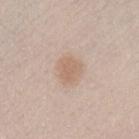Findings:
* follow-up: imaged on a skin check; not biopsied
* patient: male, aged approximately 40
* imaging modality: ~15 mm tile from a whole-body skin photo
* anatomic site: the chest
* illumination: white-light illumination
* lesion diameter: ≈3 mm